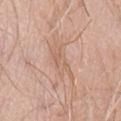follow-up: total-body-photography surveillance lesion; no biopsy | anatomic site: the abdomen | patient: female, aged approximately 60 | imaging modality: ~15 mm tile from a whole-body skin photo.